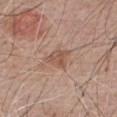TBP lesion metrics = an area of roughly 5.5 mm², an eccentricity of roughly 0.6, and a symmetry-axis asymmetry near 0.35; a peripheral color-asymmetry measure near 1; a nevus-likeness score of about 5/100
site = the chest
patient = male, roughly 65 years of age
tile lighting = white-light
diameter = ≈3 mm
acquisition = total-body-photography crop, ~15 mm field of view